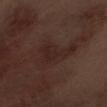Clinical impression: The lesion was photographed on a routine skin check and not biopsied; there is no pathology result. Acquisition and patient details: This is a white-light tile. Cropped from a total-body skin-imaging series; the visible field is about 15 mm. The recorded lesion diameter is about 5.5 mm. From the right forearm. A male patient in their 70s.